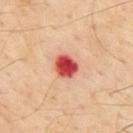{"biopsy_status": "not biopsied; imaged during a skin examination", "image": {"source": "total-body photography crop", "field_of_view_mm": 15}, "lesion_size": {"long_diameter_mm_approx": 3.0}, "site": "back", "patient": {"sex": "male", "age_approx": 55}}